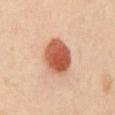<record>
  <biopsy_status>not biopsied; imaged during a skin examination</biopsy_status>
  <site>front of the torso</site>
  <image>
    <source>total-body photography crop</source>
    <field_of_view_mm>15</field_of_view_mm>
  </image>
  <lighting>cross-polarized</lighting>
  <lesion_size>
    <long_diameter_mm_approx>4.5</long_diameter_mm_approx>
  </lesion_size>
  <patient>
    <sex>female</sex>
    <age_approx>55</age_approx>
  </patient>
</record>